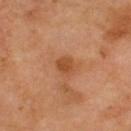Clinical impression:
This lesion was catalogued during total-body skin photography and was not selected for biopsy.
Context:
The lesion is on the upper back. Cropped from a whole-body photographic skin survey; the tile spans about 15 mm. Imaged with cross-polarized lighting. A male subject, about 70 years old. Automated image analysis of the tile measured a footprint of about 4 mm², an outline eccentricity of about 0.6 (0 = round, 1 = elongated), and two-axis asymmetry of about 0.25. And it measured a classifier nevus-likeness of about 35/100 and a detector confidence of about 100 out of 100 that the crop contains a lesion. The lesion's longest dimension is about 2.5 mm.